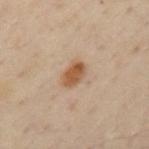Automated tile analysis of the lesion measured an area of roughly 6 mm². The software also gave border irregularity of about 1.5 on a 0–10 scale and a within-lesion color-variation index near 3/10. And it measured a nevus-likeness score of about 100/100 and a detector confidence of about 100 out of 100 that the crop contains a lesion. Cropped from a whole-body photographic skin survey; the tile spans about 15 mm. Approximately 3.5 mm at its widest. A male subject aged 43–47. The lesion is on the left upper arm.